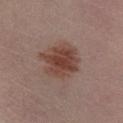follow-up = no biopsy performed (imaged during a skin exam)
lighting = cross-polarized illumination
lesion size = about 4.5 mm
body site = the left lower leg
imaging modality = ~15 mm tile from a whole-body skin photo
subject = male, about 40 years old